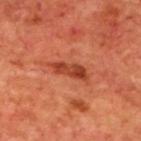A 15 mm crop from a total-body photograph taken for skin-cancer surveillance. Automated tile analysis of the lesion measured a footprint of about 6.5 mm², a shape eccentricity near 0.95, and two-axis asymmetry of about 0.35. The analysis additionally found a border-irregularity index near 4/10, a within-lesion color-variation index near 4.5/10, and radial color variation of about 1.5. It also reported a classifier nevus-likeness of about 30/100 and a lesion-detection confidence of about 100/100. Captured under cross-polarized illumination. A male subject approximately 70 years of age. The lesion is located on the upper back.